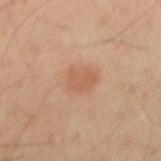No biopsy was performed on this lesion — it was imaged during a full skin examination and was not determined to be concerning. A male patient, approximately 50 years of age. On the right upper arm. A 15 mm close-up tile from a total-body photography series done for melanoma screening. Measured at roughly 4.5 mm in maximum diameter. The tile uses cross-polarized illumination. An algorithmic analysis of the crop reported a mean CIELAB color near L≈52 a*≈19 b*≈29, a lesion–skin lightness drop of about 6, and a normalized border contrast of about 4.5. The software also gave lesion-presence confidence of about 100/100.